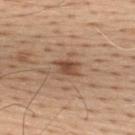Acquisition and patient details: A male patient, in their mid- to late 50s. The lesion is located on the upper back. A roughly 15 mm field-of-view crop from a total-body skin photograph.